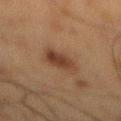No biopsy was performed on this lesion — it was imaged during a full skin examination and was not determined to be concerning.
Automated tile analysis of the lesion measured a lesion color around L≈31 a*≈16 b*≈24 in CIELAB, about 8 CIELAB-L* units darker than the surrounding skin, and a normalized lesion–skin contrast near 8. It also reported border irregularity of about 3 on a 0–10 scale, a within-lesion color-variation index near 3.5/10, and a peripheral color-asymmetry measure near 1.5. And it measured a classifier nevus-likeness of about 90/100 and a lesion-detection confidence of about 100/100.
This is a cross-polarized tile.
The recorded lesion diameter is about 4.5 mm.
A male subject, roughly 60 years of age.
A close-up tile cropped from a whole-body skin photograph, about 15 mm across.
The lesion is located on the mid back.